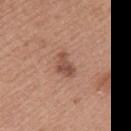location: the left upper arm | image-analysis metrics: a footprint of about 4.5 mm², an outline eccentricity of about 0.8 (0 = round, 1 = elongated), and two-axis asymmetry of about 0.35; a classifier nevus-likeness of about 50/100 and lesion-presence confidence of about 100/100 | image: ~15 mm tile from a whole-body skin photo | tile lighting: white-light illumination | subject: female, roughly 40 years of age | lesion diameter: about 3 mm.